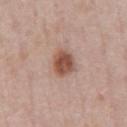– biopsy status · catalogued during a skin exam; not biopsied
– size · about 3.5 mm
– patient · male, about 50 years old
– body site · the chest
– image source · 15 mm crop, total-body photography
– illumination · white-light illumination